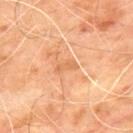Recorded during total-body skin imaging; not selected for excision or biopsy.
The lesion is on the back.
Cropped from a total-body skin-imaging series; the visible field is about 15 mm.
A male subject, in their mid- to late 60s.